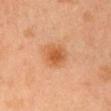Assessment:
Part of a total-body skin-imaging series; this lesion was reviewed on a skin check and was not flagged for biopsy.
Context:
About 3.5 mm across. This is a cross-polarized tile. This image is a 15 mm lesion crop taken from a total-body photograph. The lesion is located on the head or neck. A female patient, aged 18 to 22.